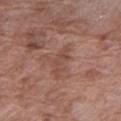Captured during whole-body skin photography for melanoma surveillance; the lesion was not biopsied.
A female subject roughly 70 years of age.
A region of skin cropped from a whole-body photographic capture, roughly 15 mm wide.
On the arm.